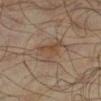<record>
<biopsy_status>not biopsied; imaged during a skin examination</biopsy_status>
<patient>
  <sex>male</sex>
  <age_approx>65</age_approx>
</patient>
<automated_metrics>
  <cielab_L>44</cielab_L>
  <cielab_a>15</cielab_a>
  <cielab_b>26</cielab_b>
  <vs_skin_darker_L>6.0</vs_skin_darker_L>
  <vs_skin_contrast_norm>5.5</vs_skin_contrast_norm>
  <peripheral_color_asymmetry>1.5</peripheral_color_asymmetry>
  <nevus_likeness_0_100>0</nevus_likeness_0_100>
  <lesion_detection_confidence_0_100>100</lesion_detection_confidence_0_100>
</automated_metrics>
<lesion_size>
  <long_diameter_mm_approx>4.0</long_diameter_mm_approx>
</lesion_size>
<image>
  <source>total-body photography crop</source>
  <field_of_view_mm>15</field_of_view_mm>
</image>
<site>right lower leg</site>
</record>